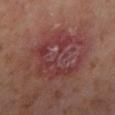Recorded during total-body skin imaging; not selected for excision or biopsy. A 15 mm close-up extracted from a 3D total-body photography capture. Located on the leg. Imaged with cross-polarized lighting. Automated image analysis of the tile measured an area of roughly 28 mm², a shape eccentricity near 0.6, and two-axis asymmetry of about 0.4. And it measured a nevus-likeness score of about 0/100 and a detector confidence of about 100 out of 100 that the crop contains a lesion. A female patient in their mid-50s. Longest diameter approximately 7 mm.